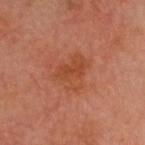Q: Was this lesion biopsied?
A: no biopsy performed (imaged during a skin exam)
Q: What lighting was used for the tile?
A: cross-polarized
Q: What are the patient's age and sex?
A: male, aged 58 to 62
Q: What is the lesion's diameter?
A: about 4 mm
Q: What is the anatomic site?
A: the head or neck
Q: What is the imaging modality?
A: 15 mm crop, total-body photography